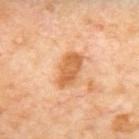Recorded during total-body skin imaging; not selected for excision or biopsy.
The lesion's longest dimension is about 4.5 mm.
A 15 mm crop from a total-body photograph taken for skin-cancer surveillance.
An algorithmic analysis of the crop reported a symmetry-axis asymmetry near 0.25. The software also gave a lesion–skin lightness drop of about 12 and a lesion-to-skin contrast of about 8.5 (normalized; higher = more distinct). The analysis additionally found a border-irregularity rating of about 2.5/10 and internal color variation of about 2.5 on a 0–10 scale. It also reported a nevus-likeness score of about 65/100 and a lesion-detection confidence of about 100/100.
Located on the back.
A female subject, about 50 years old.